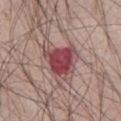Acquisition and patient details:
A male subject, approximately 65 years of age. The total-body-photography lesion software estimated a mean CIELAB color near L≈47 a*≈26 b*≈19 and a normalized lesion–skin contrast near 10. The recorded lesion diameter is about 6 mm. The lesion is located on the abdomen. Cropped from a whole-body photographic skin survey; the tile spans about 15 mm. Imaged with white-light lighting.